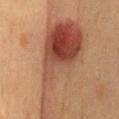Recorded during total-body skin imaging; not selected for excision or biopsy.
Located on the abdomen.
Captured under cross-polarized illumination.
A female patient aged 38–42.
The total-body-photography lesion software estimated an area of roughly 41 mm², an outline eccentricity of about 0.95 (0 = round, 1 = elongated), and a shape-asymmetry score of about 0.65 (0 = symmetric). It also reported a lesion–skin lightness drop of about 12 and a normalized lesion–skin contrast near 10. It also reported a classifier nevus-likeness of about 100/100 and a lesion-detection confidence of about 100/100.
A roughly 15 mm field-of-view crop from a total-body skin photograph.